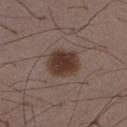{
  "biopsy_status": "not biopsied; imaged during a skin examination",
  "lesion_size": {
    "long_diameter_mm_approx": 4.0
  },
  "automated_metrics": {
    "nevus_likeness_0_100": 95,
    "lesion_detection_confidence_0_100": 100
  },
  "patient": {
    "sex": "male",
    "age_approx": 50
  },
  "site": "abdomen",
  "lighting": "white-light",
  "image": {
    "source": "total-body photography crop",
    "field_of_view_mm": 15
  }
}